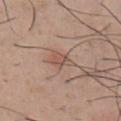Clinical impression:
Imaged during a routine full-body skin examination; the lesion was not biopsied and no histopathology is available.
Background:
A male subject in their 30s. The lesion is on the chest. A region of skin cropped from a whole-body photographic capture, roughly 15 mm wide.